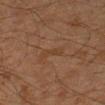• biopsy status: total-body-photography surveillance lesion; no biopsy
• anatomic site: the right forearm
• size: ~2.5 mm (longest diameter)
• image: total-body-photography crop, ~15 mm field of view
• patient: male, aged 43 to 47
• tile lighting: cross-polarized